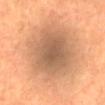No biopsy was performed on this lesion — it was imaged during a full skin examination and was not determined to be concerning. A 15 mm close-up extracted from a 3D total-body photography capture. Located on the front of the torso. A male patient aged 63 to 67.